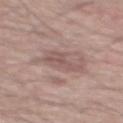This lesion was catalogued during total-body skin photography and was not selected for biopsy.